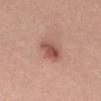Clinical impression:
This lesion was catalogued during total-body skin photography and was not selected for biopsy.
Acquisition and patient details:
Located on the mid back. A female patient, aged around 35. A region of skin cropped from a whole-body photographic capture, roughly 15 mm wide. Automated tile analysis of the lesion measured an automated nevus-likeness rating near 85 out of 100 and a lesion-detection confidence of about 100/100.